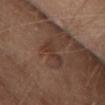workup: no biopsy performed (imaged during a skin exam)
lesion diameter: about 4.5 mm
image: ~15 mm crop, total-body skin-cancer survey
site: the front of the torso
lighting: white-light
patient: female, aged 48 to 52